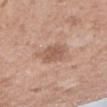notes = catalogued during a skin exam; not biopsied
image source = 15 mm crop, total-body photography
subject = female, aged approximately 40
TBP lesion metrics = border irregularity of about 2.5 on a 0–10 scale, a within-lesion color-variation index near 2.5/10, and a peripheral color-asymmetry measure near 1
tile lighting = white-light illumination
diameter = ≈3.5 mm
location = the right upper arm